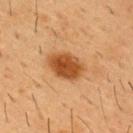Recorded during total-body skin imaging; not selected for excision or biopsy. Cropped from a whole-body photographic skin survey; the tile spans about 15 mm. The recorded lesion diameter is about 4.5 mm. Located on the front of the torso. This is a cross-polarized tile. The subject is a male aged around 55.